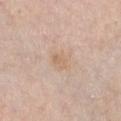Assessment: Recorded during total-body skin imaging; not selected for excision or biopsy. Clinical summary: On the chest. This is a white-light tile. The subject is a female aged 63 to 67. A close-up tile cropped from a whole-body skin photograph, about 15 mm across. About 2.5 mm across.